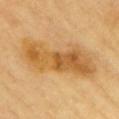biopsy_status: not biopsied; imaged during a skin examination
patient:
  sex: male
  age_approx: 60
image:
  source: total-body photography crop
  field_of_view_mm: 15
site: front of the torso
automated_metrics:
  eccentricity: 0.95
  shape_asymmetry: 0.35
  nevus_likeness_0_100: 30
lesion_size:
  long_diameter_mm_approx: 9.5
lighting: cross-polarized